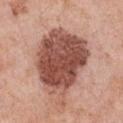subject = female, aged approximately 40; imaging modality = ~15 mm tile from a whole-body skin photo; anatomic site = the chest; lesion size = ≈9 mm; tile lighting = white-light.